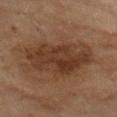body site = the left upper arm | subject = female, aged 58–62 | image = ~15 mm tile from a whole-body skin photo | tile lighting = cross-polarized | automated metrics = a lesion color around L≈33 a*≈17 b*≈27 in CIELAB, a lesion–skin lightness drop of about 9, and a normalized border contrast of about 8.5; a nevus-likeness score of about 15/100 and a lesion-detection confidence of about 100/100.